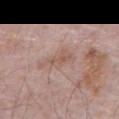<tbp_lesion>
  <biopsy_status>not biopsied; imaged during a skin examination</biopsy_status>
  <site>abdomen</site>
  <image>
    <source>total-body photography crop</source>
    <field_of_view_mm>15</field_of_view_mm>
  </image>
  <lesion_size>
    <long_diameter_mm_approx>4.0</long_diameter_mm_approx>
  </lesion_size>
  <patient>
    <sex>male</sex>
    <age_approx>70</age_approx>
  </patient>
  <lighting>white-light</lighting>
</tbp_lesion>